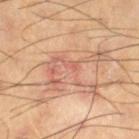follow-up = total-body-photography surveillance lesion; no biopsy
patient = male, about 70 years old
image source = ~15 mm crop, total-body skin-cancer survey
site = the right thigh
TBP lesion metrics = border irregularity of about 10 on a 0–10 scale, internal color variation of about 6 on a 0–10 scale, and radial color variation of about 2
lesion diameter = ≈7.5 mm
illumination = cross-polarized